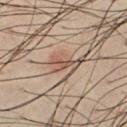Assessment: Part of a total-body skin-imaging series; this lesion was reviewed on a skin check and was not flagged for biopsy. Clinical summary: The total-body-photography lesion software estimated an eccentricity of roughly 0.9 and two-axis asymmetry of about 0.6. It also reported a classifier nevus-likeness of about 55/100 and a detector confidence of about 95 out of 100 that the crop contains a lesion. A roughly 15 mm field-of-view crop from a total-body skin photograph. The lesion is on the chest. About 2.5 mm across. A male patient aged 48–52. The tile uses cross-polarized illumination.